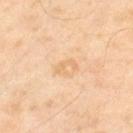Imaged during a routine full-body skin examination; the lesion was not biopsied and no histopathology is available. Cropped from a whole-body photographic skin survey; the tile spans about 15 mm. This is a cross-polarized tile. About 2.5 mm across. The subject is a male aged approximately 55. On the left thigh.